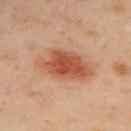biopsy status: catalogued during a skin exam; not biopsied.